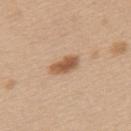workup=no biopsy performed (imaged during a skin exam) | TBP lesion metrics=a symmetry-axis asymmetry near 0.2; an average lesion color of about L≈57 a*≈20 b*≈34 (CIELAB), a lesion–skin lightness drop of about 13, and a normalized border contrast of about 8.5; border irregularity of about 2.5 on a 0–10 scale and a color-variation rating of about 3.5/10; a classifier nevus-likeness of about 95/100 | diameter=~4 mm (longest diameter) | acquisition=~15 mm tile from a whole-body skin photo | illumination=white-light illumination | location=the upper back | subject=female, in their 40s.